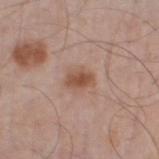{"biopsy_status": "not biopsied; imaged during a skin examination", "automated_metrics": {"cielab_L": 52, "cielab_a": 21, "cielab_b": 29, "vs_skin_darker_L": 10.0, "border_irregularity_0_10": 2.0, "color_variation_0_10": 2.5, "peripheral_color_asymmetry": 1.0, "lesion_detection_confidence_0_100": 100}, "patient": {"sex": "male", "age_approx": 60}, "lighting": "white-light", "lesion_size": {"long_diameter_mm_approx": 3.0}, "site": "left thigh", "image": {"source": "total-body photography crop", "field_of_view_mm": 15}}